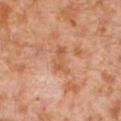Assessment:
Imaged during a routine full-body skin examination; the lesion was not biopsied and no histopathology is available.
Clinical summary:
A male subject, aged approximately 30. Approximately 3.5 mm at its widest. The tile uses cross-polarized illumination. A lesion tile, about 15 mm wide, cut from a 3D total-body photograph. Located on the leg.